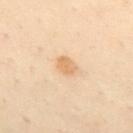Clinical summary:
Automated image analysis of the tile measured a lesion area of about 4 mm², an outline eccentricity of about 0.75 (0 = round, 1 = elongated), and a shape-asymmetry score of about 0.15 (0 = symmetric). It also reported a mean CIELAB color near L≈62 a*≈16 b*≈35, a lesion–skin lightness drop of about 8, and a lesion-to-skin contrast of about 6.5 (normalized; higher = more distinct). It also reported a color-variation rating of about 2/10 and a peripheral color-asymmetry measure near 0.5. The lesion's longest dimension is about 2.5 mm. The subject is a female aged 58 to 62. A close-up tile cropped from a whole-body skin photograph, about 15 mm across. The lesion is on the front of the torso.